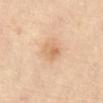Assessment:
Part of a total-body skin-imaging series; this lesion was reviewed on a skin check and was not flagged for biopsy.
Context:
A 15 mm crop from a total-body photograph taken for skin-cancer surveillance. On the abdomen.